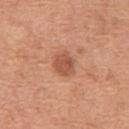No biopsy was performed on this lesion — it was imaged during a full skin examination and was not determined to be concerning.
The patient is a male aged around 65.
Automated image analysis of the tile measured a footprint of about 6 mm² and a symmetry-axis asymmetry near 0.2. It also reported internal color variation of about 2.5 on a 0–10 scale and radial color variation of about 1. The analysis additionally found an automated nevus-likeness rating near 80 out of 100.
Longest diameter approximately 2.5 mm.
A 15 mm close-up extracted from a 3D total-body photography capture.
The tile uses white-light illumination.
From the front of the torso.